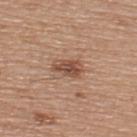Assessment: The lesion was photographed on a routine skin check and not biopsied; there is no pathology result. Background: A male patient aged approximately 65. Cropped from a total-body skin-imaging series; the visible field is about 15 mm. The lesion's longest dimension is about 3.5 mm. From the upper back. This is a white-light tile.